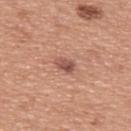The lesion was tiled from a total-body skin photograph and was not biopsied.
The total-body-photography lesion software estimated an average lesion color of about L≈53 a*≈24 b*≈27 (CIELAB), about 11 CIELAB-L* units darker than the surrounding skin, and a normalized lesion–skin contrast near 7.5. It also reported border irregularity of about 3 on a 0–10 scale and radial color variation of about 1.5. It also reported a nevus-likeness score of about 85/100 and a detector confidence of about 100 out of 100 that the crop contains a lesion.
The subject is a male roughly 50 years of age.
Approximately 2.5 mm at its widest.
Imaged with white-light lighting.
A roughly 15 mm field-of-view crop from a total-body skin photograph.
Located on the upper back.